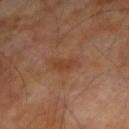Imaged during a routine full-body skin examination; the lesion was not biopsied and no histopathology is available. Located on the right forearm. A roughly 15 mm field-of-view crop from a total-body skin photograph. Measured at roughly 2.5 mm in maximum diameter. Automated image analysis of the tile measured a color-variation rating of about 0/10 and a peripheral color-asymmetry measure near 0. The software also gave an automated nevus-likeness rating near 5 out of 100 and a lesion-detection confidence of about 100/100. A male subject, roughly 70 years of age. This is a cross-polarized tile.